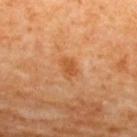Imaged during a routine full-body skin examination; the lesion was not biopsied and no histopathology is available. An algorithmic analysis of the crop reported a footprint of about 4.5 mm², a shape eccentricity near 0.65, and two-axis asymmetry of about 0.25. And it measured a color-variation rating of about 1.5/10 and a peripheral color-asymmetry measure near 0.5. About 2.5 mm across. From the upper back. Cropped from a whole-body photographic skin survey; the tile spans about 15 mm. The patient is a female in their mid-60s.